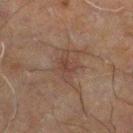A region of skin cropped from a whole-body photographic capture, roughly 15 mm wide.
The total-body-photography lesion software estimated an area of roughly 6.5 mm², an eccentricity of roughly 0.8, and two-axis asymmetry of about 0.25. It also reported an average lesion color of about L≈31 a*≈14 b*≈20 (CIELAB) and a normalized lesion–skin contrast near 5.
The recorded lesion diameter is about 4.5 mm.
Located on the leg.
This is a cross-polarized tile.
A male patient aged 58 to 62.